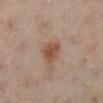The lesion was photographed on a routine skin check and not biopsied; there is no pathology result.
A female subject aged approximately 40.
This is a cross-polarized tile.
Cropped from a total-body skin-imaging series; the visible field is about 15 mm.
From the leg.